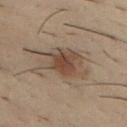workup — total-body-photography surveillance lesion; no biopsy
body site — the chest
subject — male, aged approximately 30
lighting — cross-polarized
automated lesion analysis — a lesion area of about 19 mm², an eccentricity of roughly 0.65, and a symmetry-axis asymmetry near 0.25; a lesion color around L≈37 a*≈11 b*≈21 in CIELAB, roughly 8 lightness units darker than nearby skin, and a normalized lesion–skin contrast near 7
acquisition — ~15 mm crop, total-body skin-cancer survey
diameter — ~6 mm (longest diameter)